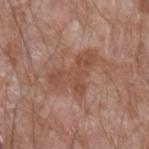biopsy_status: not biopsied; imaged during a skin examination
image:
  source: total-body photography crop
  field_of_view_mm: 15
patient:
  sex: male
  age_approx: 60
lighting: white-light
site: left forearm
lesion_size:
  long_diameter_mm_approx: 6.0